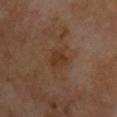Case summary:
- size: ~3 mm (longest diameter)
- tile lighting: cross-polarized
- automated metrics: a lesion color around L≈31 a*≈18 b*≈27 in CIELAB, roughly 6 lightness units darker than nearby skin, and a lesion-to-skin contrast of about 6.5 (normalized; higher = more distinct); a border-irregularity index near 3/10, a within-lesion color-variation index near 1.5/10, and a peripheral color-asymmetry measure near 0.5
- imaging modality: ~15 mm tile from a whole-body skin photo
- subject: male, roughly 55 years of age
- location: the chest